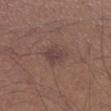This lesion was catalogued during total-body skin photography and was not selected for biopsy. A male subject, in their mid- to late 60s. On the right lower leg. A region of skin cropped from a whole-body photographic capture, roughly 15 mm wide.